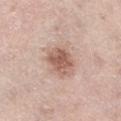Notes:
* biopsy status: total-body-photography surveillance lesion; no biopsy
* patient: female, in their mid- to late 60s
* TBP lesion metrics: a mean CIELAB color near L≈58 a*≈20 b*≈27, a lesion–skin lightness drop of about 13, and a normalized lesion–skin contrast near 8.5; border irregularity of about 2 on a 0–10 scale and radial color variation of about 1.5; an automated nevus-likeness rating near 50 out of 100 and a detector confidence of about 100 out of 100 that the crop contains a lesion
* site: the leg
* tile lighting: white-light illumination
* acquisition: ~15 mm tile from a whole-body skin photo
* diameter: about 3.5 mm